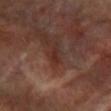Imaged during a routine full-body skin examination; the lesion was not biopsied and no histopathology is available. The lesion is located on the arm. The subject is a female roughly 60 years of age. A roughly 15 mm field-of-view crop from a total-body skin photograph.